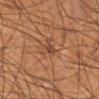Impression: Captured during whole-body skin photography for melanoma surveillance; the lesion was not biopsied. Image and clinical context: The patient is a male in their mid-50s. The lesion is on the right lower leg. This is a cross-polarized tile. A close-up tile cropped from a whole-body skin photograph, about 15 mm across. Measured at roughly 2.5 mm in maximum diameter.